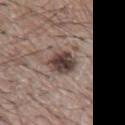Findings:
• workup: total-body-photography surveillance lesion; no biopsy
• automated lesion analysis: a border-irregularity index near 1.5/10 and a peripheral color-asymmetry measure near 1.5; lesion-presence confidence of about 100/100
• location: the mid back
• patient: male, in their mid-60s
• image source: total-body-photography crop, ~15 mm field of view
• lighting: white-light
• lesion diameter: about 3.5 mm